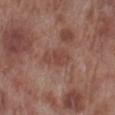Part of a total-body skin-imaging series; this lesion was reviewed on a skin check and was not flagged for biopsy. The lesion is on the right lower leg. The tile uses white-light illumination. Measured at roughly 4 mm in maximum diameter. A 15 mm close-up tile from a total-body photography series done for melanoma screening. A male patient in their 70s.